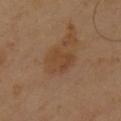{
  "biopsy_status": "not biopsied; imaged during a skin examination"
}